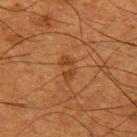Case summary:
- follow-up · imaged on a skin check; not biopsied
- patient · male, aged around 50
- image · total-body-photography crop, ~15 mm field of view
- site · the left upper arm
- lighting · cross-polarized illumination